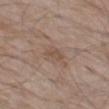follow-up: imaged on a skin check; not biopsied
lighting: white-light illumination
size: ~2.5 mm (longest diameter)
image: ~15 mm tile from a whole-body skin photo
location: the left thigh
subject: male, aged 58 to 62